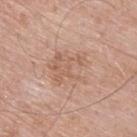biopsy status=catalogued during a skin exam; not biopsied | patient=male, aged around 65 | automated metrics=an area of roughly 17 mm², an eccentricity of roughly 0.3, and a symmetry-axis asymmetry near 0.2; a normalized border contrast of about 4.5; a nevus-likeness score of about 0/100 and a lesion-detection confidence of about 100/100 | image source=15 mm crop, total-body photography | lighting=white-light illumination | body site=the upper back.